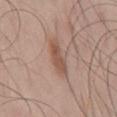Notes:
* follow-up — total-body-photography surveillance lesion; no biopsy
* imaging modality — total-body-photography crop, ~15 mm field of view
* anatomic site — the mid back
* subject — male, about 45 years old